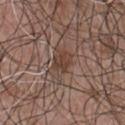Captured during whole-body skin photography for melanoma surveillance; the lesion was not biopsied. Cropped from a total-body skin-imaging series; the visible field is about 15 mm. The lesion's longest dimension is about 3 mm. A male subject roughly 50 years of age. The lesion is located on the front of the torso. Imaged with white-light lighting. Automated image analysis of the tile measured a lesion area of about 5 mm², an eccentricity of roughly 0.7, and a shape-asymmetry score of about 0.2 (0 = symmetric). The analysis additionally found a classifier nevus-likeness of about 0/100 and a detector confidence of about 95 out of 100 that the crop contains a lesion.